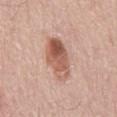Imaged during a routine full-body skin examination; the lesion was not biopsied and no histopathology is available.